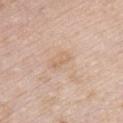Q: Was a biopsy performed?
A: catalogued during a skin exam; not biopsied
Q: What kind of image is this?
A: total-body-photography crop, ~15 mm field of view
Q: Automated lesion metrics?
A: an average lesion color of about L≈65 a*≈17 b*≈33 (CIELAB) and a normalized border contrast of about 5
Q: What are the patient's age and sex?
A: male, aged approximately 40
Q: How was the tile lit?
A: white-light
Q: Where on the body is the lesion?
A: the chest
Q: How large is the lesion?
A: about 2.5 mm